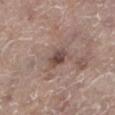Clinical impression:
Part of a total-body skin-imaging series; this lesion was reviewed on a skin check and was not flagged for biopsy.
Context:
A close-up tile cropped from a whole-body skin photograph, about 15 mm across. The subject is a female roughly 85 years of age or older. This is a white-light tile. The lesion-visualizer software estimated an average lesion color of about L≈46 a*≈17 b*≈21 (CIELAB), about 11 CIELAB-L* units darker than the surrounding skin, and a normalized lesion–skin contrast near 8. Located on the left lower leg. The lesion's longest dimension is about 2.5 mm.